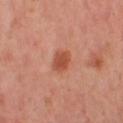| key | value |
|---|---|
| workup | total-body-photography surveillance lesion; no biopsy |
| illumination | cross-polarized illumination |
| subject | female, aged around 40 |
| diameter | ≈3 mm |
| location | the right leg |
| imaging modality | ~15 mm crop, total-body skin-cancer survey |